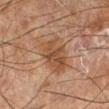Imaged during a routine full-body skin examination; the lesion was not biopsied and no histopathology is available. The recorded lesion diameter is about 5.5 mm. On the right lower leg. Automated image analysis of the tile measured an area of roughly 14 mm². And it measured a mean CIELAB color near L≈42 a*≈18 b*≈29 and a normalized lesion–skin contrast near 7. The software also gave a border-irregularity index near 3/10 and peripheral color asymmetry of about 1.5. The tile uses cross-polarized illumination. A male subject aged around 65. This image is a 15 mm lesion crop taken from a total-body photograph.